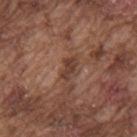Recorded during total-body skin imaging; not selected for excision or biopsy. Imaged with white-light lighting. A male patient, approximately 75 years of age. Measured at roughly 2.5 mm in maximum diameter. The lesion is on the upper back. Cropped from a total-body skin-imaging series; the visible field is about 15 mm.